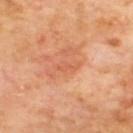Findings:
– workup: no biopsy performed (imaged during a skin exam)
– acquisition: 15 mm crop, total-body photography
– patient: male, about 70 years old
– site: the back
– size: about 3 mm
– illumination: cross-polarized illumination